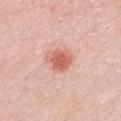| key | value |
|---|---|
| biopsy status | no biopsy performed (imaged during a skin exam) |
| lesion size | ≈3 mm |
| location | the chest |
| subject | female, in their 40s |
| acquisition | 15 mm crop, total-body photography |